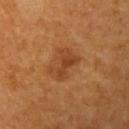| field | value |
|---|---|
| notes | catalogued during a skin exam; not biopsied |
| tile lighting | cross-polarized illumination |
| acquisition | total-body-photography crop, ~15 mm field of view |
| lesion diameter | ≈4 mm |
| patient | roughly 70 years of age |
| image-analysis metrics | a shape eccentricity near 0.75 and a symmetry-axis asymmetry near 0.3; a mean CIELAB color near L≈43 a*≈24 b*≈38, roughly 8 lightness units darker than nearby skin, and a lesion-to-skin contrast of about 6.5 (normalized; higher = more distinct); a border-irregularity index near 3.5/10, internal color variation of about 3.5 on a 0–10 scale, and peripheral color asymmetry of about 1; a classifier nevus-likeness of about 45/100 |
| anatomic site | the arm |